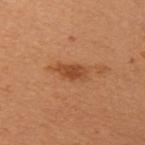workup: imaged on a skin check; not biopsied | lighting: cross-polarized illumination | body site: the left upper arm | patient: female, aged around 50 | lesion size: ~3 mm (longest diameter) | image: ~15 mm crop, total-body skin-cancer survey | TBP lesion metrics: a mean CIELAB color near L≈39 a*≈22 b*≈32 and about 9 CIELAB-L* units darker than the surrounding skin; a classifier nevus-likeness of about 70/100 and a lesion-detection confidence of about 100/100.